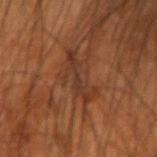lesion size = ~5.5 mm (longest diameter) | subject = male, aged 53–57 | anatomic site = the arm | imaging modality = ~15 mm crop, total-body skin-cancer survey | automated lesion analysis = a shape eccentricity near 0.95 and a symmetry-axis asymmetry near 0.55; a lesion color around L≈32 a*≈20 b*≈28 in CIELAB, a lesion–skin lightness drop of about 6, and a normalized border contrast of about 6.5; a border-irregularity index near 9/10, a within-lesion color-variation index near 0.5/10, and a peripheral color-asymmetry measure near 0; a nevus-likeness score of about 0/100 and a lesion-detection confidence of about 65/100.